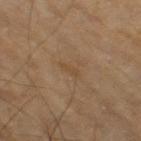Q: Was this lesion biopsied?
A: imaged on a skin check; not biopsied